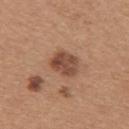Captured during whole-body skin photography for melanoma surveillance; the lesion was not biopsied.
Longest diameter approximately 3.5 mm.
On the upper back.
A 15 mm close-up tile from a total-body photography series done for melanoma screening.
Automated tile analysis of the lesion measured an area of roughly 8.5 mm² and a shape-asymmetry score of about 0.15 (0 = symmetric). The analysis additionally found border irregularity of about 1.5 on a 0–10 scale and a color-variation rating of about 4/10. The software also gave an automated nevus-likeness rating near 15 out of 100 and lesion-presence confidence of about 100/100.
Imaged with white-light lighting.
The subject is a female aged around 30.